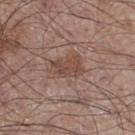follow-up: no biopsy performed (imaged during a skin exam) | TBP lesion metrics: a lesion area of about 7 mm²; internal color variation of about 3.5 on a 0–10 scale and radial color variation of about 1; a lesion-detection confidence of about 100/100 | location: the right thigh | patient: male, roughly 60 years of age | acquisition: ~15 mm tile from a whole-body skin photo.